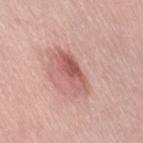site: right thigh
image:
  source: total-body photography crop
  field_of_view_mm: 15
patient:
  sex: female
  age_approx: 65